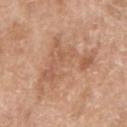<tbp_lesion>
<biopsy_status>not biopsied; imaged during a skin examination</biopsy_status>
<image>
  <source>total-body photography crop</source>
  <field_of_view_mm>15</field_of_view_mm>
</image>
<patient>
  <sex>male</sex>
  <age_approx>60</age_approx>
</patient>
<automated_metrics>
  <area_mm2_approx>24.0</area_mm2_approx>
  <border_irregularity_0_10>10.0</border_irregularity_0_10>
</automated_metrics>
<site>right upper arm</site>
<lesion_size>
  <long_diameter_mm_approx>8.5</long_diameter_mm_approx>
</lesion_size>
</tbp_lesion>